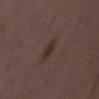biopsy status: imaged on a skin check; not biopsied | patient: female, roughly 35 years of age | anatomic site: the back | image-analysis metrics: two-axis asymmetry of about 0.2; a lesion-detection confidence of about 100/100 | image source: ~15 mm tile from a whole-body skin photo | illumination: white-light illumination | lesion diameter: about 3 mm.